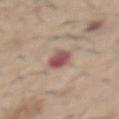Acquisition and patient details: From the abdomen. A lesion tile, about 15 mm wide, cut from a 3D total-body photograph. The total-body-photography lesion software estimated a mean CIELAB color near L≈52 a*≈24 b*≈22 and about 14 CIELAB-L* units darker than the surrounding skin. A male subject, aged 58 to 62. The tile uses white-light illumination.